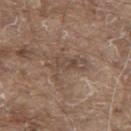Clinical impression:
Imaged during a routine full-body skin examination; the lesion was not biopsied and no histopathology is available.
Image and clinical context:
An algorithmic analysis of the crop reported a mean CIELAB color near L≈47 a*≈15 b*≈24. And it measured a border-irregularity rating of about 7.5/10 and peripheral color asymmetry of about 1.5. A male patient approximately 80 years of age. Located on the upper back. A region of skin cropped from a whole-body photographic capture, roughly 15 mm wide. This is a white-light tile. Approximately 4.5 mm at its widest.